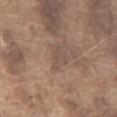The lesion was tiled from a total-body skin photograph and was not biopsied. Longest diameter approximately 3 mm. A male patient about 75 years old. Imaged with white-light lighting. An algorithmic analysis of the crop reported an area of roughly 3.5 mm², an eccentricity of roughly 0.8, and a shape-asymmetry score of about 0.6 (0 = symmetric). It also reported a lesion color around L≈50 a*≈15 b*≈23 in CIELAB and a normalized lesion–skin contrast near 5. The analysis additionally found a color-variation rating of about 0/10. A roughly 15 mm field-of-view crop from a total-body skin photograph. The lesion is located on the chest.